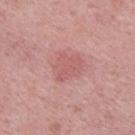Impression: Imaged during a routine full-body skin examination; the lesion was not biopsied and no histopathology is available. Context: A 15 mm close-up extracted from a 3D total-body photography capture. A male subject roughly 50 years of age. This is a white-light tile. Located on the upper back. The lesion's longest dimension is about 4 mm.